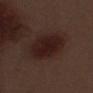Part of a total-body skin-imaging series; this lesion was reviewed on a skin check and was not flagged for biopsy. The lesion is on the left thigh. The lesion-visualizer software estimated a shape eccentricity near 0.75 and a shape-asymmetry score of about 0.15 (0 = symmetric). The software also gave roughly 8 lightness units darker than nearby skin. The patient is a male in their 70s. A lesion tile, about 15 mm wide, cut from a 3D total-body photograph. The tile uses white-light illumination.